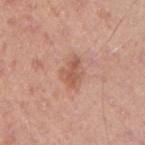Part of a total-body skin-imaging series; this lesion was reviewed on a skin check and was not flagged for biopsy. The patient is a male aged 38 to 42. On the arm. Approximately 3 mm at its widest. A roughly 15 mm field-of-view crop from a total-body skin photograph. Captured under white-light illumination.